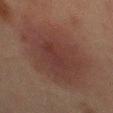* notes: total-body-photography surveillance lesion; no biopsy
* image source: ~15 mm tile from a whole-body skin photo
* lighting: cross-polarized illumination
* anatomic site: the abdomen
* patient: male, in their mid- to late 60s